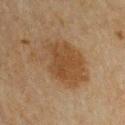Q: Was a biopsy performed?
A: catalogued during a skin exam; not biopsied
Q: Lesion location?
A: the arm
Q: Patient demographics?
A: male, approximately 65 years of age
Q: What is the lesion's diameter?
A: ≈7.5 mm
Q: What lighting was used for the tile?
A: cross-polarized
Q: How was this image acquired?
A: ~15 mm tile from a whole-body skin photo